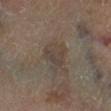{"biopsy_status": "not biopsied; imaged during a skin examination", "patient": {"sex": "male", "age_approx": 70}, "lighting": "cross-polarized", "site": "right lower leg", "image": {"source": "total-body photography crop", "field_of_view_mm": 15}}